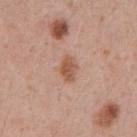Q: Was this lesion biopsied?
A: catalogued during a skin exam; not biopsied
Q: Where on the body is the lesion?
A: the abdomen
Q: How large is the lesion?
A: ≈3 mm
Q: Patient demographics?
A: male, aged 53 to 57
Q: What lighting was used for the tile?
A: white-light
Q: What is the imaging modality?
A: total-body-photography crop, ~15 mm field of view
Q: Automated lesion metrics?
A: a classifier nevus-likeness of about 85/100 and a lesion-detection confidence of about 100/100